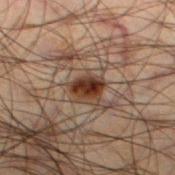The lesion was tiled from a total-body skin photograph and was not biopsied. On the left thigh. The recorded lesion diameter is about 3 mm. The total-body-photography lesion software estimated a border-irregularity rating of about 1.5/10, internal color variation of about 4.5 on a 0–10 scale, and peripheral color asymmetry of about 1.5. The software also gave a classifier nevus-likeness of about 95/100 and a detector confidence of about 100 out of 100 that the crop contains a lesion. Captured under cross-polarized illumination. The patient is a male aged around 50. A lesion tile, about 15 mm wide, cut from a 3D total-body photograph.